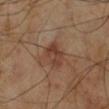Q: Was a biopsy performed?
A: catalogued during a skin exam; not biopsied
Q: How was this image acquired?
A: ~15 mm crop, total-body skin-cancer survey
Q: Lesion location?
A: the right lower leg
Q: Who is the patient?
A: male, about 70 years old